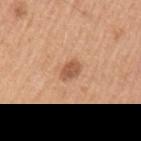follow-up — catalogued during a skin exam; not biopsied
acquisition — total-body-photography crop, ~15 mm field of view
subject — male, aged around 50
site — the left upper arm